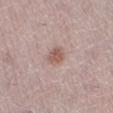Impression: This lesion was catalogued during total-body skin photography and was not selected for biopsy. Background: A 15 mm crop from a total-body photograph taken for skin-cancer surveillance. A female patient, roughly 50 years of age. The tile uses white-light illumination. The lesion's longest dimension is about 3 mm. The lesion is on the right lower leg.